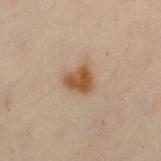<case>
<biopsy_status>not biopsied; imaged during a skin examination</biopsy_status>
<patient>
  <sex>female</sex>
  <age_approx>50</age_approx>
</patient>
<automated_metrics>
  <area_mm2_approx>7.0</area_mm2_approx>
  <shape_asymmetry>0.35</shape_asymmetry>
  <cielab_L>45</cielab_L>
  <cielab_a>16</cielab_a>
  <cielab_b>30</cielab_b>
  <vs_skin_darker_L>10.0</vs_skin_darker_L>
  <border_irregularity_0_10>3.0</border_irregularity_0_10>
  <color_variation_0_10>3.0</color_variation_0_10>
  <peripheral_color_asymmetry>1.0</peripheral_color_asymmetry>
</automated_metrics>
<image>
  <source>total-body photography crop</source>
  <field_of_view_mm>15</field_of_view_mm>
</image>
<lighting>cross-polarized</lighting>
<lesion_size>
  <long_diameter_mm_approx>3.0</long_diameter_mm_approx>
</lesion_size>
<site>leg</site>
</case>